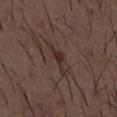The lesion was photographed on a routine skin check and not biopsied; there is no pathology result.
Approximately 4 mm at its widest.
A male subject approximately 50 years of age.
From the mid back.
The lesion-visualizer software estimated a border-irregularity rating of about 6.5/10, internal color variation of about 1 on a 0–10 scale, and a peripheral color-asymmetry measure near 0.5. The software also gave a classifier nevus-likeness of about 10/100 and a lesion-detection confidence of about 100/100.
A 15 mm close-up tile from a total-body photography series done for melanoma screening.